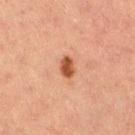Impression: The lesion was tiled from a total-body skin photograph and was not biopsied.